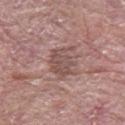Case summary:
– biopsy status — imaged on a skin check; not biopsied
– subject — male, roughly 60 years of age
– site — the arm
– lighting — white-light
– acquisition — ~15 mm tile from a whole-body skin photo
– automated lesion analysis — a lesion color around L≈51 a*≈19 b*≈22 in CIELAB, a lesion–skin lightness drop of about 9, and a lesion-to-skin contrast of about 6.5 (normalized; higher = more distinct); a border-irregularity rating of about 4.5/10, a color-variation rating of about 3/10, and a peripheral color-asymmetry measure near 1
– size — about 4.5 mm